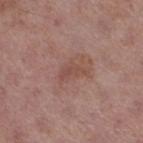Findings:
* workup — total-body-photography surveillance lesion; no biopsy
* anatomic site — the right thigh
* size — about 3 mm
* acquisition — ~15 mm tile from a whole-body skin photo
* subject — male, in their 70s